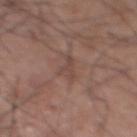Notes:
– follow-up · total-body-photography surveillance lesion; no biopsy
– body site · the abdomen
– image source · ~15 mm crop, total-body skin-cancer survey
– lighting · white-light illumination
– diameter · ≈3.5 mm
– subject · male, aged 53 to 57
– automated lesion analysis · a lesion area of about 4 mm², an outline eccentricity of about 0.9 (0 = round, 1 = elongated), and a symmetry-axis asymmetry near 0.55; border irregularity of about 6.5 on a 0–10 scale, a within-lesion color-variation index near 0.5/10, and a peripheral color-asymmetry measure near 0; a classifier nevus-likeness of about 0/100 and a detector confidence of about 75 out of 100 that the crop contains a lesion